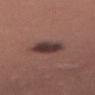<lesion>
  <biopsy_status>not biopsied; imaged during a skin examination</biopsy_status>
  <lighting>white-light</lighting>
  <site>right thigh</site>
  <lesion_size>
    <long_diameter_mm_approx>4.0</long_diameter_mm_approx>
  </lesion_size>
  <image>
    <source>total-body photography crop</source>
    <field_of_view_mm>15</field_of_view_mm>
  </image>
  <patient>
    <sex>female</sex>
    <age_approx>35</age_approx>
  </patient>
</lesion>